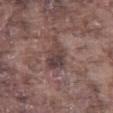Clinical impression: This lesion was catalogued during total-body skin photography and was not selected for biopsy. Context: A lesion tile, about 15 mm wide, cut from a 3D total-body photograph. Longest diameter approximately 4.5 mm. A male subject, about 75 years old. On the right thigh.